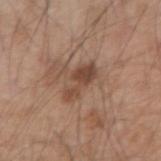| key | value |
|---|---|
| diameter | ~4.5 mm (longest diameter) |
| acquisition | 15 mm crop, total-body photography |
| subject | male, aged 53–57 |
| anatomic site | the arm |
| TBP lesion metrics | a footprint of about 7 mm² and a shape-asymmetry score of about 0.45 (0 = symmetric); an average lesion color of about L≈46 a*≈19 b*≈28 (CIELAB), a lesion–skin lightness drop of about 10, and a normalized border contrast of about 7.5 |
| lighting | white-light illumination |